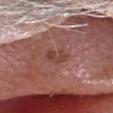{"biopsy_status": "not biopsied; imaged during a skin examination", "image": {"source": "total-body photography crop", "field_of_view_mm": 15}, "site": "head or neck", "patient": {"sex": "male", "age_approx": 80}}